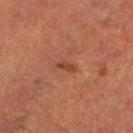Q: Was this lesion biopsied?
A: no biopsy performed (imaged during a skin exam)